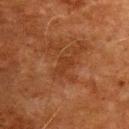Notes:
• biopsy status: total-body-photography surveillance lesion; no biopsy
• lesion diameter: about 2.5 mm
• acquisition: 15 mm crop, total-body photography
• subject: male, approximately 80 years of age
• site: the front of the torso